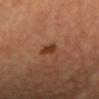The lesion was photographed on a routine skin check and not biopsied; there is no pathology result. The lesion is located on the head or neck. Imaged with cross-polarized lighting. The recorded lesion diameter is about 2.5 mm. A 15 mm close-up extracted from a 3D total-body photography capture. The patient is a female aged around 40.